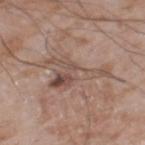  biopsy_status: not biopsied; imaged during a skin examination
  patient:
    sex: male
    age_approx: 60
  site: left thigh
  image:
    source: total-body photography crop
    field_of_view_mm: 15